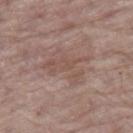Notes:
- acquisition — ~15 mm tile from a whole-body skin photo
- site — the right thigh
- size — ~5 mm (longest diameter)
- patient — female, about 85 years old or older